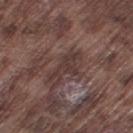{"biopsy_status": "not biopsied; imaged during a skin examination", "lesion_size": {"long_diameter_mm_approx": 5.0}, "lighting": "white-light", "image": {"source": "total-body photography crop", "field_of_view_mm": 15}, "automated_metrics": {"border_irregularity_0_10": 6.0, "color_variation_0_10": 1.5, "peripheral_color_asymmetry": 0.5}, "site": "right thigh", "patient": {"sex": "male", "age_approx": 75}}